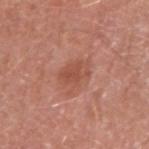Part of a total-body skin-imaging series; this lesion was reviewed on a skin check and was not flagged for biopsy. Located on the right upper arm. A 15 mm crop from a total-body photograph taken for skin-cancer surveillance. The subject is a male approximately 70 years of age. The total-body-photography lesion software estimated a detector confidence of about 100 out of 100 that the crop contains a lesion. The recorded lesion diameter is about 4 mm.